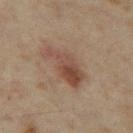follow-up: catalogued during a skin exam; not biopsied.